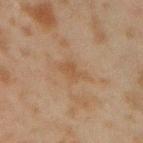biopsy status: imaged on a skin check; not biopsied | site: the right forearm | subject: male, roughly 45 years of age | illumination: cross-polarized illumination | imaging modality: ~15 mm crop, total-body skin-cancer survey | diameter: ~3.5 mm (longest diameter).